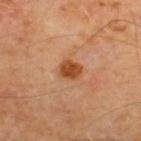Part of a total-body skin-imaging series; this lesion was reviewed on a skin check and was not flagged for biopsy. A 15 mm crop from a total-body photograph taken for skin-cancer surveillance. A male patient aged around 65. The lesion is on the upper back. The recorded lesion diameter is about 3 mm. An algorithmic analysis of the crop reported a lesion area of about 5.5 mm², an eccentricity of roughly 0.6, and a symmetry-axis asymmetry near 0.15. It also reported an average lesion color of about L≈48 a*≈26 b*≈39 (CIELAB) and about 12 CIELAB-L* units darker than the surrounding skin. And it measured a classifier nevus-likeness of about 90/100. This is a cross-polarized tile.